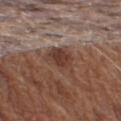Case summary:
– workup — total-body-photography surveillance lesion; no biopsy
– tile lighting — white-light
– location — the left upper arm
– subject — male, aged 73–77
– automated metrics — a border-irregularity rating of about 4/10 and radial color variation of about 1; a classifier nevus-likeness of about 0/100
– size — ≈4 mm
– acquisition — 15 mm crop, total-body photography